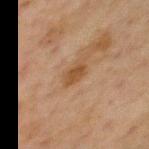Notes:
- imaging modality · ~15 mm crop, total-body skin-cancer survey
- tile lighting · cross-polarized
- body site · the chest
- patient · male, about 75 years old